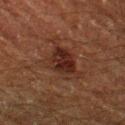biopsy status — total-body-photography surveillance lesion; no biopsy
illumination — cross-polarized illumination
automated lesion analysis — an area of roughly 14 mm², an outline eccentricity of about 0.85 (0 = round, 1 = elongated), and a shape-asymmetry score of about 0.35 (0 = symmetric); an average lesion color of about L≈21 a*≈17 b*≈20 (CIELAB), about 7 CIELAB-L* units darker than the surrounding skin, and a normalized lesion–skin contrast near 8.5; a border-irregularity index near 5/10, internal color variation of about 4.5 on a 0–10 scale, and radial color variation of about 1.5; a classifier nevus-likeness of about 85/100 and a lesion-detection confidence of about 100/100
patient — male, aged 58 to 62
acquisition — total-body-photography crop, ~15 mm field of view
size — ~6.5 mm (longest diameter)
anatomic site — the left thigh